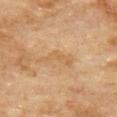Clinical impression:
Captured during whole-body skin photography for melanoma surveillance; the lesion was not biopsied.
Background:
On the upper back. A region of skin cropped from a whole-body photographic capture, roughly 15 mm wide. A male subject about 70 years old.